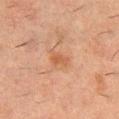Recorded during total-body skin imaging; not selected for excision or biopsy. The lesion's longest dimension is about 2.5 mm. The tile uses cross-polarized illumination. From the chest. A roughly 15 mm field-of-view crop from a total-body skin photograph. A male patient, aged approximately 50.